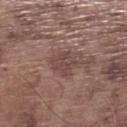Q: Is there a histopathology result?
A: imaged on a skin check; not biopsied
Q: Who is the patient?
A: male, in their mid- to late 70s
Q: What kind of image is this?
A: total-body-photography crop, ~15 mm field of view
Q: What is the anatomic site?
A: the left lower leg